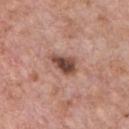Case summary:
* notes — imaged on a skin check; not biopsied
* lesion diameter — ~4 mm (longest diameter)
* tile lighting — white-light
* imaging modality — ~15 mm tile from a whole-body skin photo
* anatomic site — the chest
* subject — male, aged 58 to 62
* image-analysis metrics — an average lesion color of about L≈48 a*≈21 b*≈26 (CIELAB), about 15 CIELAB-L* units darker than the surrounding skin, and a normalized lesion–skin contrast near 10.5